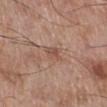This lesion was catalogued during total-body skin photography and was not selected for biopsy.
A male subject, aged approximately 70.
The lesion is located on the right lower leg.
A 15 mm close-up tile from a total-body photography series done for melanoma screening.